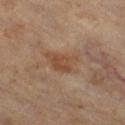workup: total-body-photography surveillance lesion; no biopsy | anatomic site: the right thigh | image: total-body-photography crop, ~15 mm field of view | patient: male, in their 60s | lighting: cross-polarized illumination | automated metrics: an eccentricity of roughly 0.8 and a shape-asymmetry score of about 0.35 (0 = symmetric); a mean CIELAB color near L≈43 a*≈18 b*≈29 and a lesion-to-skin contrast of about 6.5 (normalized; higher = more distinct); border irregularity of about 4 on a 0–10 scale and a color-variation rating of about 4/10 | lesion size: ~4 mm (longest diameter).